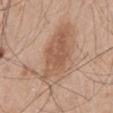Assessment: Part of a total-body skin-imaging series; this lesion was reviewed on a skin check and was not flagged for biopsy. Context: A lesion tile, about 15 mm wide, cut from a 3D total-body photograph. Longest diameter approximately 9.5 mm. Imaged with white-light lighting. The patient is a male aged 43 to 47. The lesion is on the mid back. The total-body-photography lesion software estimated an eccentricity of roughly 0.8 and a shape-asymmetry score of about 0.4 (0 = symmetric). It also reported a border-irregularity index near 6/10 and internal color variation of about 5 on a 0–10 scale. The analysis additionally found lesion-presence confidence of about 100/100.